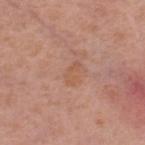Impression:
Recorded during total-body skin imaging; not selected for excision or biopsy.
Background:
Imaged with white-light lighting. Located on the right thigh. Automated image analysis of the tile measured a mean CIELAB color near L≈56 a*≈22 b*≈32, roughly 5 lightness units darker than nearby skin, and a normalized border contrast of about 5.5. And it measured a classifier nevus-likeness of about 0/100 and a detector confidence of about 100 out of 100 that the crop contains a lesion. The lesion's longest dimension is about 2.5 mm. A female patient aged around 55. A 15 mm crop from a total-body photograph taken for skin-cancer surveillance.